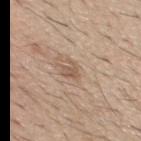| feature | finding |
|---|---|
| image-analysis metrics | an average lesion color of about L≈56 a*≈16 b*≈28 (CIELAB), a lesion–skin lightness drop of about 9, and a lesion-to-skin contrast of about 6 (normalized; higher = more distinct); border irregularity of about 4.5 on a 0–10 scale and internal color variation of about 3 on a 0–10 scale |
| subject | male, aged 38 to 42 |
| lighting | white-light |
| site | the mid back |
| acquisition | ~15 mm tile from a whole-body skin photo |
| lesion diameter | ~2.5 mm (longest diameter) |